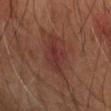No biopsy was performed on this lesion — it was imaged during a full skin examination and was not determined to be concerning.
Automated tile analysis of the lesion measured a lesion area of about 9 mm², an outline eccentricity of about 0.75 (0 = round, 1 = elongated), and a shape-asymmetry score of about 0.3 (0 = symmetric). It also reported a mean CIELAB color near L≈33 a*≈24 b*≈23. The analysis additionally found internal color variation of about 4 on a 0–10 scale and peripheral color asymmetry of about 1.5.
This image is a 15 mm lesion crop taken from a total-body photograph.
Located on the right forearm.
A male patient, aged 68–72.
This is a cross-polarized tile.